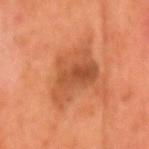{
  "biopsy_status": "not biopsied; imaged during a skin examination",
  "site": "head or neck",
  "patient": {
    "sex": "male",
    "age_approx": 55
  },
  "image": {
    "source": "total-body photography crop",
    "field_of_view_mm": 15
  }
}